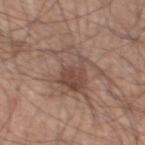{"biopsy_status": "not biopsied; imaged during a skin examination", "lighting": "white-light", "automated_metrics": {"cielab_L": 48, "cielab_a": 17, "cielab_b": 24, "vs_skin_darker_L": 9.0, "vs_skin_contrast_norm": 6.5, "border_irregularity_0_10": 8.5, "color_variation_0_10": 6.5, "peripheral_color_asymmetry": 2.5, "nevus_likeness_0_100": 15}, "site": "left thigh", "lesion_size": {"long_diameter_mm_approx": 8.0}, "image": {"source": "total-body photography crop", "field_of_view_mm": 15}, "patient": {"sex": "male", "age_approx": 60}}